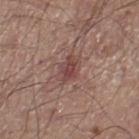No biopsy was performed on this lesion — it was imaged during a full skin examination and was not determined to be concerning. This image is a 15 mm lesion crop taken from a total-body photograph. The lesion is located on the right lower leg. A male patient, aged around 55. This is a white-light tile.